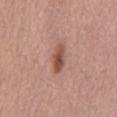biopsy status: total-body-photography surveillance lesion; no biopsy | acquisition: 15 mm crop, total-body photography | TBP lesion metrics: a shape eccentricity near 0.9; roughly 12 lightness units darker than nearby skin; a border-irregularity index near 2.5/10, a within-lesion color-variation index near 5/10, and peripheral color asymmetry of about 1.5; a classifier nevus-likeness of about 90/100 and a lesion-detection confidence of about 100/100 | tile lighting: white-light illumination | patient: male, about 55 years old.